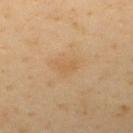The lesion was tiled from a total-body skin photograph and was not biopsied.
A female subject, aged 48 to 52.
Automated tile analysis of the lesion measured an average lesion color of about L≈59 a*≈17 b*≈38 (CIELAB), roughly 5 lightness units darker than nearby skin, and a normalized border contrast of about 4.5. The analysis additionally found border irregularity of about 3.5 on a 0–10 scale and a peripheral color-asymmetry measure near 1.
The recorded lesion diameter is about 3 mm.
Captured under cross-polarized illumination.
Cropped from a total-body skin-imaging series; the visible field is about 15 mm.
On the upper back.